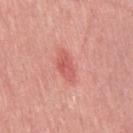Q: What lighting was used for the tile?
A: white-light illumination
Q: Who is the patient?
A: female, aged 38 to 42
Q: What is the imaging modality?
A: ~15 mm tile from a whole-body skin photo
Q: What is the lesion's diameter?
A: ~3.5 mm (longest diameter)
Q: What is the anatomic site?
A: the right thigh